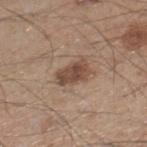Impression: Imaged during a routine full-body skin examination; the lesion was not biopsied and no histopathology is available. Context: About 4 mm across. The lesion is on the right lower leg. The patient is a male aged around 45. A close-up tile cropped from a whole-body skin photograph, about 15 mm across.